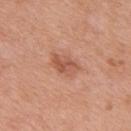notes: total-body-photography surveillance lesion; no biopsy | location: the mid back | patient: male, aged 68 to 72 | tile lighting: white-light illumination | lesion size: about 4 mm | imaging modality: ~15 mm crop, total-body skin-cancer survey.